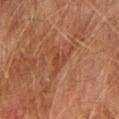Q: Was this lesion biopsied?
A: catalogued during a skin exam; not biopsied
Q: Automated lesion metrics?
A: about 5 CIELAB-L* units darker than the surrounding skin and a normalized lesion–skin contrast near 4.5
Q: What is the imaging modality?
A: ~15 mm tile from a whole-body skin photo
Q: Lesion location?
A: the right upper arm
Q: Patient demographics?
A: male, in their mid-70s
Q: Illumination type?
A: cross-polarized illumination